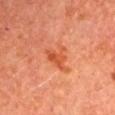Part of a total-body skin-imaging series; this lesion was reviewed on a skin check and was not flagged for biopsy. The lesion is on the arm. Approximately 3.5 mm at its widest. The tile uses cross-polarized illumination. The patient is a male aged 63–67. This image is a 15 mm lesion crop taken from a total-body photograph.